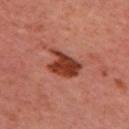Q: Is there a histopathology result?
A: no biopsy performed (imaged during a skin exam)
Q: How was this image acquired?
A: total-body-photography crop, ~15 mm field of view
Q: What are the patient's age and sex?
A: male, in their mid-60s
Q: Lesion location?
A: the upper back